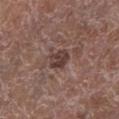Findings:
* notes · imaged on a skin check; not biopsied
* subject · male, in their mid-70s
* tile lighting · white-light
* acquisition · ~15 mm tile from a whole-body skin photo
* site · the left lower leg